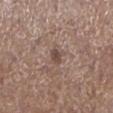location: the leg
image: ~15 mm crop, total-body skin-cancer survey
image-analysis metrics: a lesion area of about 3 mm², an outline eccentricity of about 0.55 (0 = round, 1 = elongated), and two-axis asymmetry of about 0.3; a mean CIELAB color near L≈46 a*≈16 b*≈22, roughly 9 lightness units darker than nearby skin, and a normalized lesion–skin contrast near 7; a border-irregularity rating of about 2.5/10, a within-lesion color-variation index near 1.5/10, and peripheral color asymmetry of about 0.5
subject: male, aged approximately 75